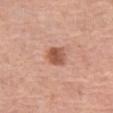Assessment:
Recorded during total-body skin imaging; not selected for excision or biopsy.
Background:
Cropped from a total-body skin-imaging series; the visible field is about 15 mm. The recorded lesion diameter is about 3 mm. Located on the left thigh. Captured under white-light illumination. The patient is a female aged 63–67.